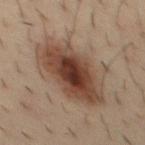{
  "biopsy_status": "not biopsied; imaged during a skin examination",
  "patient": {
    "sex": "male",
    "age_approx": 35
  },
  "lesion_size": {
    "long_diameter_mm_approx": 9.0
  },
  "site": "chest",
  "image": {
    "source": "total-body photography crop",
    "field_of_view_mm": 15
  },
  "lighting": "cross-polarized"
}